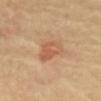notes — no biopsy performed (imaged during a skin exam)
illumination — cross-polarized
anatomic site — the front of the torso
image source — 15 mm crop, total-body photography
TBP lesion metrics — an area of roughly 7 mm², an outline eccentricity of about 0.7 (0 = round, 1 = elongated), and two-axis asymmetry of about 0.3; an average lesion color of about L≈58 a*≈23 b*≈34 (CIELAB), a lesion–skin lightness drop of about 8, and a normalized border contrast of about 5.5; border irregularity of about 3 on a 0–10 scale, a color-variation rating of about 4.5/10, and a peripheral color-asymmetry measure near 1.5
patient — female, in their mid-60s